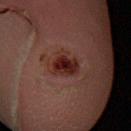Clinical impression:
Recorded during total-body skin imaging; not selected for excision or biopsy.
Clinical summary:
A male patient aged around 60. Imaged with cross-polarized lighting. Cropped from a total-body skin-imaging series; the visible field is about 15 mm. The lesion is on the left forearm. An algorithmic analysis of the crop reported a mean CIELAB color near L≈24 a*≈22 b*≈21 and roughly 9 lightness units darker than nearby skin. The analysis additionally found a classifier nevus-likeness of about 60/100 and a detector confidence of about 100 out of 100 that the crop contains a lesion.